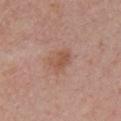Cropped from a whole-body photographic skin survey; the tile spans about 15 mm. The patient is a female in their mid- to late 50s. Located on the chest.